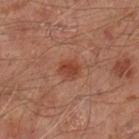Impression:
Part of a total-body skin-imaging series; this lesion was reviewed on a skin check and was not flagged for biopsy.
Context:
The lesion is on the left lower leg. Captured under cross-polarized illumination. Automated tile analysis of the lesion measured roughly 9 lightness units darker than nearby skin and a lesion-to-skin contrast of about 7.5 (normalized; higher = more distinct). And it measured a nevus-likeness score of about 80/100. Measured at roughly 2.5 mm in maximum diameter. A male subject, roughly 65 years of age. Cropped from a total-body skin-imaging series; the visible field is about 15 mm.